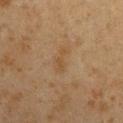  biopsy_status: not biopsied; imaged during a skin examination